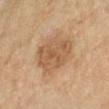Findings:
- workup · imaged on a skin check; not biopsied
- subject · female, approximately 60 years of age
- lesion diameter · ~5.5 mm (longest diameter)
- automated lesion analysis · a lesion area of about 18 mm², an eccentricity of roughly 0.6, and two-axis asymmetry of about 0.15; an average lesion color of about L≈58 a*≈19 b*≈36 (CIELAB) and about 10 CIELAB-L* units darker than the surrounding skin; a border-irregularity index near 2/10, a color-variation rating of about 3.5/10, and radial color variation of about 1
- location · the left forearm
- acquisition · total-body-photography crop, ~15 mm field of view